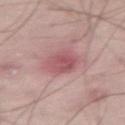Q: Was a biopsy performed?
A: total-body-photography surveillance lesion; no biopsy
Q: What kind of image is this?
A: ~15 mm crop, total-body skin-cancer survey
Q: Lesion location?
A: the abdomen
Q: Patient demographics?
A: male, aged approximately 65
Q: What lighting was used for the tile?
A: white-light illumination